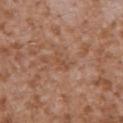Impression: Captured during whole-body skin photography for melanoma surveillance; the lesion was not biopsied. Clinical summary: Longest diameter approximately 2.5 mm. A lesion tile, about 15 mm wide, cut from a 3D total-body photograph. A male patient, aged 43–47. Imaged with white-light lighting. The lesion is on the left upper arm.